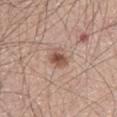Captured during whole-body skin photography for melanoma surveillance; the lesion was not biopsied. Imaged with white-light lighting. A 15 mm close-up extracted from a 3D total-body photography capture. A male subject approximately 65 years of age. The lesion is on the abdomen. The lesion-visualizer software estimated an average lesion color of about L≈53 a*≈20 b*≈26 (CIELAB), roughly 12 lightness units darker than nearby skin, and a normalized lesion–skin contrast near 8. The software also gave a color-variation rating of about 5/10 and peripheral color asymmetry of about 1. And it measured a lesion-detection confidence of about 100/100.